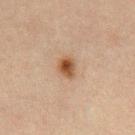Q: Is there a histopathology result?
A: total-body-photography surveillance lesion; no biopsy
Q: Who is the patient?
A: male, aged 48–52
Q: How was this image acquired?
A: ~15 mm crop, total-body skin-cancer survey
Q: Lesion location?
A: the abdomen